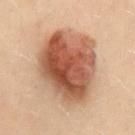The lesion was tiled from a total-body skin photograph and was not biopsied. From the lower back. A roughly 15 mm field-of-view crop from a total-body skin photograph. The tile uses cross-polarized illumination. The patient is a female aged around 30. Approximately 9 mm at its widest.